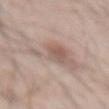Assessment: Imaged during a routine full-body skin examination; the lesion was not biopsied and no histopathology is available. Context: The lesion is located on the abdomen. A male subject roughly 65 years of age. Cropped from a total-body skin-imaging series; the visible field is about 15 mm.